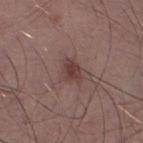Recorded during total-body skin imaging; not selected for excision or biopsy.
A male patient roughly 35 years of age.
The lesion is on the leg.
A close-up tile cropped from a whole-body skin photograph, about 15 mm across.